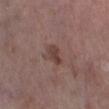Clinical impression: Part of a total-body skin-imaging series; this lesion was reviewed on a skin check and was not flagged for biopsy. Clinical summary: On the left lower leg. The recorded lesion diameter is about 3 mm. A female subject aged approximately 85 or older. This is a white-light tile. A roughly 15 mm field-of-view crop from a total-body skin photograph.